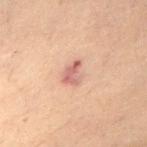This lesion was catalogued during total-body skin photography and was not selected for biopsy.
On the lower back.
Measured at roughly 3 mm in maximum diameter.
Automated image analysis of the tile measured a footprint of about 5 mm² and a shape-asymmetry score of about 0.3 (0 = symmetric). The software also gave a classifier nevus-likeness of about 0/100.
A 15 mm crop from a total-body photograph taken for skin-cancer surveillance.
Captured under cross-polarized illumination.
A female subject roughly 80 years of age.